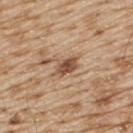Q: What is the imaging modality?
A: total-body-photography crop, ~15 mm field of view
Q: How was the tile lit?
A: white-light
Q: Patient demographics?
A: male, aged around 70
Q: Where on the body is the lesion?
A: the upper back